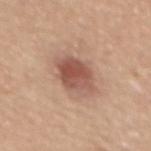Clinical impression:
This lesion was catalogued during total-body skin photography and was not selected for biopsy.
Background:
A close-up tile cropped from a whole-body skin photograph, about 15 mm across. The recorded lesion diameter is about 4.5 mm. A female subject, aged 53–57. The tile uses white-light illumination. From the mid back. The lesion-visualizer software estimated an eccentricity of roughly 0.7 and a shape-asymmetry score of about 0.15 (0 = symmetric). The software also gave an average lesion color of about L≈54 a*≈22 b*≈27 (CIELAB), a lesion–skin lightness drop of about 12, and a lesion-to-skin contrast of about 8 (normalized; higher = more distinct).